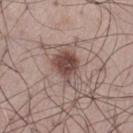Recorded during total-body skin imaging; not selected for excision or biopsy. The lesion is on the left thigh. Imaged with white-light lighting. The lesion's longest dimension is about 5 mm. A lesion tile, about 15 mm wide, cut from a 3D total-body photograph. A male patient approximately 60 years of age.